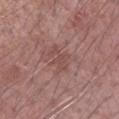Imaged during a routine full-body skin examination; the lesion was not biopsied and no histopathology is available.
A male patient roughly 70 years of age.
A lesion tile, about 15 mm wide, cut from a 3D total-body photograph.
Approximately 3.5 mm at its widest.
Captured under white-light illumination.
From the left forearm.